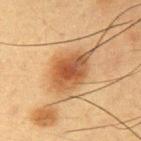Case summary:
- biopsy status: no biopsy performed (imaged during a skin exam)